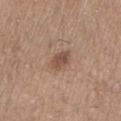biopsy status = catalogued during a skin exam; not biopsied | subject = male, in their mid-60s | body site = the right forearm | lesion diameter = about 3 mm | acquisition = 15 mm crop, total-body photography.